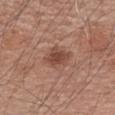Q: Where on the body is the lesion?
A: the left forearm
Q: Illumination type?
A: white-light
Q: What kind of image is this?
A: ~15 mm tile from a whole-body skin photo
Q: What are the patient's age and sex?
A: male, aged 58 to 62
Q: What is the lesion's diameter?
A: ≈4 mm